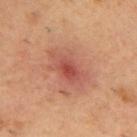  biopsy_status: not biopsied; imaged during a skin examination
  lesion_size:
    long_diameter_mm_approx: 5.5
  site: upper back
  patient:
    sex: male
    age_approx: 55
  image:
    source: total-body photography crop
    field_of_view_mm: 15
  lighting: cross-polarized